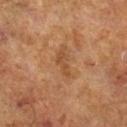Q: What is the anatomic site?
A: the right lower leg
Q: Who is the patient?
A: male, aged around 70
Q: How was the tile lit?
A: cross-polarized
Q: What is the imaging modality?
A: ~15 mm crop, total-body skin-cancer survey
Q: What did automated image analysis measure?
A: a lesion color around L≈39 a*≈18 b*≈30 in CIELAB, about 6 CIELAB-L* units darker than the surrounding skin, and a normalized lesion–skin contrast near 5.5; a border-irregularity rating of about 4.5/10, internal color variation of about 1 on a 0–10 scale, and radial color variation of about 0.5